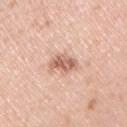  biopsy_status: not biopsied; imaged during a skin examination
  image:
    source: total-body photography crop
    field_of_view_mm: 15
  patient:
    sex: male
    age_approx: 60
  site: right upper arm